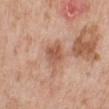The lesion was photographed on a routine skin check and not biopsied; there is no pathology result.
A roughly 15 mm field-of-view crop from a total-body skin photograph.
Located on the left lower leg.
The patient is a male aged around 80.